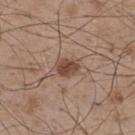A 15 mm crop from a total-body photograph taken for skin-cancer surveillance.
Longest diameter approximately 3 mm.
A male patient, aged around 50.
The lesion is located on the upper back.
Automated image analysis of the tile measured a lesion color around L≈46 a*≈18 b*≈27 in CIELAB, roughly 12 lightness units darker than nearby skin, and a normalized border contrast of about 9. The analysis additionally found a border-irregularity index near 2/10 and a within-lesion color-variation index near 3/10. It also reported a classifier nevus-likeness of about 95/100 and lesion-presence confidence of about 100/100.
Imaged with white-light lighting.